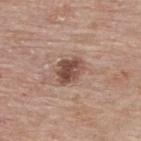Clinical summary:
Longest diameter approximately 3.5 mm. Cropped from a whole-body photographic skin survey; the tile spans about 15 mm. The subject is a male about 85 years old or older. The lesion is located on the upper back. The tile uses white-light illumination.